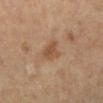lesion_size:
  long_diameter_mm_approx: 3.0
lighting: cross-polarized
patient:
  sex: female
  age_approx: 70
image:
  source: total-body photography crop
  field_of_view_mm: 15
site: left lower leg
automated_metrics:
  border_irregularity_0_10: 3.5
  color_variation_0_10: 1.5
  peripheral_color_asymmetry: 0.5
  nevus_likeness_0_100: 10
  lesion_detection_confidence_0_100: 100